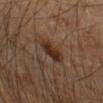The lesion was photographed on a routine skin check and not biopsied; there is no pathology result.
The lesion is located on the right forearm.
About 3.5 mm across.
The patient is a male in their 60s.
A 15 mm close-up tile from a total-body photography series done for melanoma screening.